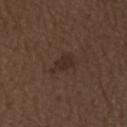{"biopsy_status": "not biopsied; imaged during a skin examination", "automated_metrics": {"cielab_L": 27, "cielab_a": 14, "cielab_b": 21, "vs_skin_darker_L": 6.0, "vs_skin_contrast_norm": 6.5}, "patient": {"sex": "male", "age_approx": 70}, "lesion_size": {"long_diameter_mm_approx": 3.0}, "site": "left forearm", "image": {"source": "total-body photography crop", "field_of_view_mm": 15}, "lighting": "white-light"}